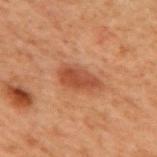biopsy status: total-body-photography surveillance lesion; no biopsy
location: the mid back
subject: male, aged 68–72
lighting: cross-polarized
lesion diameter: ~4.5 mm (longest diameter)
acquisition: ~15 mm crop, total-body skin-cancer survey